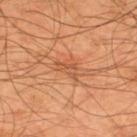follow-up: catalogued during a skin exam; not biopsied
image source: 15 mm crop, total-body photography
size: ≈3 mm
anatomic site: the upper back
patient: male, roughly 45 years of age
TBP lesion metrics: a mean CIELAB color near L≈51 a*≈25 b*≈36 and a normalized lesion–skin contrast near 5.5; a border-irregularity rating of about 5.5/10 and a color-variation rating of about 0/10; a classifier nevus-likeness of about 0/100 and a lesion-detection confidence of about 100/100
lighting: cross-polarized illumination